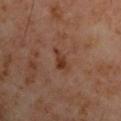Clinical impression: The lesion was tiled from a total-body skin photograph and was not biopsied. Clinical summary: A 15 mm close-up tile from a total-body photography series done for melanoma screening. A male subject, aged 58–62. The lesion is located on the left upper arm. This is a cross-polarized tile.